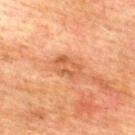The lesion was photographed on a routine skin check and not biopsied; there is no pathology result. The recorded lesion diameter is about 3.5 mm. A male patient roughly 75 years of age. Imaged with cross-polarized lighting. Cropped from a total-body skin-imaging series; the visible field is about 15 mm. Located on the upper back.